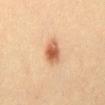follow-up: total-body-photography surveillance lesion; no biopsy | site: the abdomen | patient: male, about 40 years old | size: about 3 mm | tile lighting: cross-polarized illumination | acquisition: 15 mm crop, total-body photography.